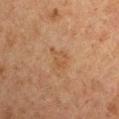Notes:
- follow-up: no biopsy performed (imaged during a skin exam)
- acquisition: ~15 mm crop, total-body skin-cancer survey
- location: the arm
- lighting: cross-polarized
- subject: male, roughly 50 years of age